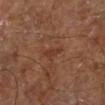Impression: Part of a total-body skin-imaging series; this lesion was reviewed on a skin check and was not flagged for biopsy.